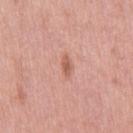The lesion was tiled from a total-body skin photograph and was not biopsied.
The subject is a female roughly 40 years of age.
The lesion is on the right thigh.
This is a white-light tile.
The recorded lesion diameter is about 2.5 mm.
A close-up tile cropped from a whole-body skin photograph, about 15 mm across.